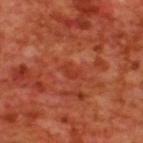The lesion's longest dimension is about 2.5 mm.
A male patient, aged approximately 70.
On the upper back.
This is a cross-polarized tile.
A lesion tile, about 15 mm wide, cut from a 3D total-body photograph.